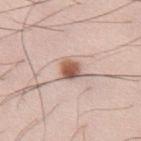Case summary:
- notes — no biopsy performed (imaged during a skin exam)
- lesion size — ~2.5 mm (longest diameter)
- patient — male, roughly 35 years of age
- body site — the abdomen
- lighting — white-light illumination
- image — ~15 mm crop, total-body skin-cancer survey